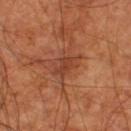Q: Was a biopsy performed?
A: imaged on a skin check; not biopsied
Q: Who is the patient?
A: male, in their 60s
Q: What lighting was used for the tile?
A: cross-polarized
Q: How was this image acquired?
A: ~15 mm tile from a whole-body skin photo
Q: Where on the body is the lesion?
A: the left thigh
Q: What is the lesion's diameter?
A: ~4 mm (longest diameter)